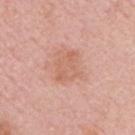  biopsy_status: not biopsied; imaged during a skin examination
  image:
    source: total-body photography crop
    field_of_view_mm: 15
  lesion_size:
    long_diameter_mm_approx: 3.5
  patient:
    sex: male
    age_approx: 65
  site: chest
  lighting: white-light